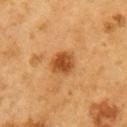Notes:
– follow-up: no biopsy performed (imaged during a skin exam)
– site: the right upper arm
– image-analysis metrics: an area of roughly 7 mm² and two-axis asymmetry of about 0.15; a mean CIELAB color near L≈51 a*≈26 b*≈43, roughly 13 lightness units darker than nearby skin, and a normalized lesion–skin contrast near 9; a border-irregularity index near 1/10, a within-lesion color-variation index near 5/10, and a peripheral color-asymmetry measure near 1.5; a classifier nevus-likeness of about 95/100 and lesion-presence confidence of about 100/100
– patient: male, roughly 60 years of age
– size: ≈3 mm
– image source: 15 mm crop, total-body photography